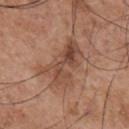follow-up = imaged on a skin check; not biopsied
lesion diameter = ~6 mm (longest diameter)
location = the chest
image = ~15 mm tile from a whole-body skin photo
patient = male, aged approximately 55